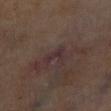This lesion was catalogued during total-body skin photography and was not selected for biopsy. A region of skin cropped from a whole-body photographic capture, roughly 15 mm wide. The patient is a male aged approximately 70. The lesion is on the right lower leg. This is a cross-polarized tile.